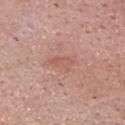{"biopsy_status": "not biopsied; imaged during a skin examination", "lesion_size": {"long_diameter_mm_approx": 3.0}, "patient": {"sex": "male", "age_approx": 70}, "site": "head or neck", "lighting": "white-light", "image": {"source": "total-body photography crop", "field_of_view_mm": 15}, "automated_metrics": {"cielab_L": 57, "cielab_a": 23, "cielab_b": 28, "vs_skin_darker_L": 6.0, "vs_skin_contrast_norm": 5.0, "nevus_likeness_0_100": 0, "lesion_detection_confidence_0_100": 100}}